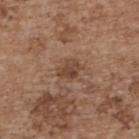Longest diameter approximately 3 mm. A female patient aged 63–67. A region of skin cropped from a whole-body photographic capture, roughly 15 mm wide. Located on the upper back. This is a white-light tile.